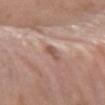{"biopsy_status": "not biopsied; imaged during a skin examination", "site": "left forearm", "automated_metrics": {"border_irregularity_0_10": 3.0, "color_variation_0_10": 0.0}, "image": {"source": "total-body photography crop", "field_of_view_mm": 15}, "lesion_size": {"long_diameter_mm_approx": 2.5}, "patient": {"sex": "male", "age_approx": 75}}